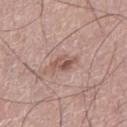{"biopsy_status": "not biopsied; imaged during a skin examination", "patient": {"sex": "male", "age_approx": 75}, "lighting": "white-light", "image": {"source": "total-body photography crop", "field_of_view_mm": 15}, "lesion_size": {"long_diameter_mm_approx": 3.0}, "site": "left thigh"}